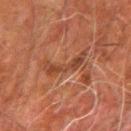From the right forearm. The subject is a male aged approximately 80. A 15 mm crop from a total-body photograph taken for skin-cancer surveillance. The lesion's longest dimension is about 4.5 mm. Imaged with cross-polarized lighting.